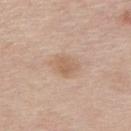Captured during whole-body skin photography for melanoma surveillance; the lesion was not biopsied.
About 3.5 mm across.
The patient is a male approximately 60 years of age.
A close-up tile cropped from a whole-body skin photograph, about 15 mm across.
The lesion is located on the back.
The lesion-visualizer software estimated an automated nevus-likeness rating near 15 out of 100 and a lesion-detection confidence of about 100/100.
This is a white-light tile.